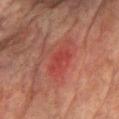Captured during whole-body skin photography for melanoma surveillance; the lesion was not biopsied.
The subject is a male in their mid-70s.
Located on the chest.
An algorithmic analysis of the crop reported a mean CIELAB color near L≈37 a*≈31 b*≈26 and a normalized border contrast of about 5.
Captured under cross-polarized illumination.
About 3.5 mm across.
A close-up tile cropped from a whole-body skin photograph, about 15 mm across.